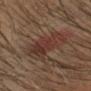workup — catalogued during a skin exam; not biopsied | automated metrics — a lesion area of about 18 mm², an eccentricity of roughly 0.45, and a shape-asymmetry score of about 0.3 (0 = symmetric); a border-irregularity index near 4.5/10, a within-lesion color-variation index near 4/10, and a peripheral color-asymmetry measure near 1; a detector confidence of about 100 out of 100 that the crop contains a lesion | diameter — about 6 mm | image source — ~15 mm tile from a whole-body skin photo | body site — the head or neck | subject — male, roughly 40 years of age.